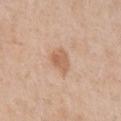Clinical impression:
Imaged during a routine full-body skin examination; the lesion was not biopsied and no histopathology is available.
Image and clinical context:
A lesion tile, about 15 mm wide, cut from a 3D total-body photograph. Located on the chest. Imaged with white-light lighting. A male subject, approximately 60 years of age. The recorded lesion diameter is about 3 mm. Automated tile analysis of the lesion measured an area of roughly 5 mm² and a shape eccentricity near 0.7. The analysis additionally found border irregularity of about 2.5 on a 0–10 scale, internal color variation of about 2.5 on a 0–10 scale, and peripheral color asymmetry of about 1. It also reported a classifier nevus-likeness of about 15/100 and a detector confidence of about 100 out of 100 that the crop contains a lesion.